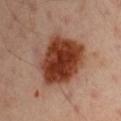Impression:
No biopsy was performed on this lesion — it was imaged during a full skin examination and was not determined to be concerning.
Background:
A 15 mm close-up extracted from a 3D total-body photography capture. This is a cross-polarized tile. About 7 mm across. A male patient aged approximately 50. On the left upper arm.